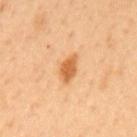subject = male, aged approximately 50 | imaging modality = 15 mm crop, total-body photography | lighting = cross-polarized illumination | diameter = ~3.5 mm (longest diameter) | anatomic site = the mid back.